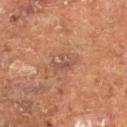Case summary:
- lesion diameter · about 2.5 mm
- automated metrics · a footprint of about 4 mm², an outline eccentricity of about 0.6 (0 = round, 1 = elongated), and two-axis asymmetry of about 0.45; roughly 6 lightness units darker than nearby skin and a normalized lesion–skin contrast near 6; internal color variation of about 1.5 on a 0–10 scale and a peripheral color-asymmetry measure near 0.5; an automated nevus-likeness rating near 0 out of 100 and a lesion-detection confidence of about 100/100
- imaging modality · ~15 mm tile from a whole-body skin photo
- tile lighting · cross-polarized illumination
- site · the right thigh
- patient · male, aged approximately 75